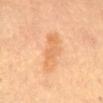Q: Was a biopsy performed?
A: imaged on a skin check; not biopsied
Q: How was this image acquired?
A: ~15 mm tile from a whole-body skin photo
Q: How large is the lesion?
A: ~6 mm (longest diameter)
Q: Who is the patient?
A: female, aged 58 to 62
Q: How was the tile lit?
A: cross-polarized illumination
Q: What is the anatomic site?
A: the chest
Q: What did automated image analysis measure?
A: a lesion area of about 10 mm² and a symmetry-axis asymmetry near 0.25; a lesion color around L≈61 a*≈21 b*≈37 in CIELAB, a lesion–skin lightness drop of about 7, and a lesion-to-skin contrast of about 5.5 (normalized; higher = more distinct)